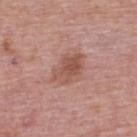{
  "biopsy_status": "not biopsied; imaged during a skin examination",
  "image": {
    "source": "total-body photography crop",
    "field_of_view_mm": 15
  },
  "lighting": "white-light",
  "patient": {
    "sex": "male",
    "age_approx": 75
  },
  "lesion_size": {
    "long_diameter_mm_approx": 4.5
  },
  "automated_metrics": {
    "cielab_L": 53,
    "cielab_a": 23,
    "cielab_b": 26,
    "vs_skin_darker_L": 9.0,
    "vs_skin_contrast_norm": 6.5,
    "border_irregularity_0_10": 3.0,
    "color_variation_0_10": 4.0,
    "peripheral_color_asymmetry": 1.5
  },
  "site": "upper back"
}